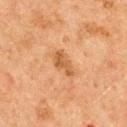Q: Is there a histopathology result?
A: no biopsy performed (imaged during a skin exam)
Q: How was this image acquired?
A: ~15 mm tile from a whole-body skin photo
Q: Lesion location?
A: the upper back
Q: What is the lesion's diameter?
A: about 3.5 mm
Q: Illumination type?
A: cross-polarized illumination
Q: Who is the patient?
A: female, about 60 years old